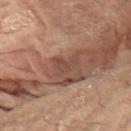Q: What is the imaging modality?
A: 15 mm crop, total-body photography
Q: Patient demographics?
A: female, aged 78–82
Q: What is the anatomic site?
A: the arm